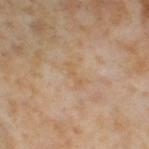Q: How was the tile lit?
A: cross-polarized illumination
Q: How was this image acquired?
A: ~15 mm crop, total-body skin-cancer survey
Q: What are the patient's age and sex?
A: female, about 55 years old
Q: Where on the body is the lesion?
A: the right thigh
Q: What did automated image analysis measure?
A: a within-lesion color-variation index near 0/10 and peripheral color asymmetry of about 0; a classifier nevus-likeness of about 0/100 and a lesion-detection confidence of about 100/100